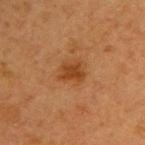Part of a total-body skin-imaging series; this lesion was reviewed on a skin check and was not flagged for biopsy. An algorithmic analysis of the crop reported an area of roughly 5 mm², a shape eccentricity near 0.65, and a shape-asymmetry score of about 0.25 (0 = symmetric). It also reported roughly 9 lightness units darker than nearby skin. The analysis additionally found border irregularity of about 2.5 on a 0–10 scale, a color-variation rating of about 2/10, and peripheral color asymmetry of about 0.5. And it measured an automated nevus-likeness rating near 70 out of 100 and a detector confidence of about 100 out of 100 that the crop contains a lesion. This image is a 15 mm lesion crop taken from a total-body photograph. Approximately 3 mm at its widest. The subject is a female approximately 40 years of age. Imaged with cross-polarized lighting. The lesion is located on the right upper arm.